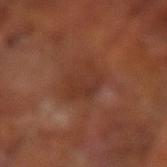This lesion was catalogued during total-body skin photography and was not selected for biopsy.